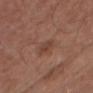{"biopsy_status": "not biopsied; imaged during a skin examination", "image": {"source": "total-body photography crop", "field_of_view_mm": 15}, "site": "head or neck", "lesion_size": {"long_diameter_mm_approx": 2.5}, "patient": {"sex": "male", "age_approx": 55}, "automated_metrics": {"cielab_L": 42, "cielab_a": 21, "cielab_b": 27, "vs_skin_darker_L": 7.0, "vs_skin_contrast_norm": 6.0, "color_variation_0_10": 3.0, "peripheral_color_asymmetry": 1.0}, "lighting": "white-light"}